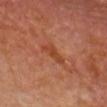The lesion was tiled from a total-body skin photograph and was not biopsied.
The lesion is located on the head or neck.
This is a cross-polarized tile.
Longest diameter approximately 3.5 mm.
The subject is a male in their mid- to late 60s.
The lesion-visualizer software estimated a classifier nevus-likeness of about 0/100 and a lesion-detection confidence of about 100/100.
A roughly 15 mm field-of-view crop from a total-body skin photograph.